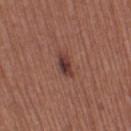On the left thigh. The recorded lesion diameter is about 3 mm. This image is a 15 mm lesion crop taken from a total-body photograph. The patient is a female aged approximately 55.